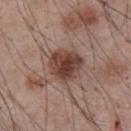About 4 mm across.
A 15 mm crop from a total-body photograph taken for skin-cancer surveillance.
Captured under white-light illumination.
A male subject aged approximately 55.
Automated image analysis of the tile measured a lesion area of about 13 mm². The analysis additionally found a border-irregularity index near 1.5/10, internal color variation of about 4.5 on a 0–10 scale, and radial color variation of about 1.5.
The lesion is located on the abdomen.